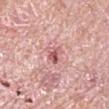Findings:
– follow-up: imaged on a skin check; not biopsied
– lighting: white-light illumination
– subject: male, about 65 years old
– image: ~15 mm tile from a whole-body skin photo
– body site: the right upper arm
– TBP lesion metrics: a footprint of about 3.5 mm², a shape eccentricity near 0.75, and a symmetry-axis asymmetry near 0.35; an average lesion color of about L≈58 a*≈28 b*≈23 (CIELAB) and a normalized border contrast of about 8; a nevus-likeness score of about 0/100